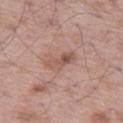- follow-up · total-body-photography surveillance lesion; no biopsy
- image-analysis metrics · a shape eccentricity near 0.9
- subject · male, approximately 50 years of age
- lighting · white-light illumination
- acquisition · ~15 mm crop, total-body skin-cancer survey
- anatomic site · the leg
- diameter · ~4 mm (longest diameter)